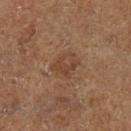<lesion>
<biopsy_status>not biopsied; imaged during a skin examination</biopsy_status>
<lesion_size>
  <long_diameter_mm_approx>3.0</long_diameter_mm_approx>
</lesion_size>
<site>left lower leg</site>
<image>
  <source>total-body photography crop</source>
  <field_of_view_mm>15</field_of_view_mm>
</image>
<lighting>cross-polarized</lighting>
<patient>
  <sex>male</sex>
  <age_approx>75</age_approx>
</patient>
<automated_metrics>
  <area_mm2_approx>6.0</area_mm2_approx>
  <eccentricity>0.65</eccentricity>
  <shape_asymmetry>0.3</shape_asymmetry>
  <nevus_likeness_0_100>0</nevus_likeness_0_100>
</automated_metrics>
</lesion>